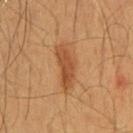Clinical impression: The lesion was tiled from a total-body skin photograph and was not biopsied. Image and clinical context: The subject is a male roughly 60 years of age. A lesion tile, about 15 mm wide, cut from a 3D total-body photograph. The lesion is on the chest.